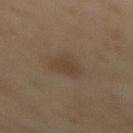biopsy status = no biopsy performed (imaged during a skin exam); illumination = cross-polarized illumination; image source = total-body-photography crop, ~15 mm field of view; subject = female, about 60 years old; location = the mid back; lesion size = ≈3.5 mm.